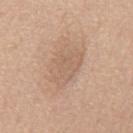A roughly 15 mm field-of-view crop from a total-body skin photograph. The patient is a male approximately 60 years of age. Located on the mid back. This is a white-light tile. The lesion's longest dimension is about 5 mm.